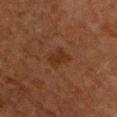This lesion was catalogued during total-body skin photography and was not selected for biopsy.
An algorithmic analysis of the crop reported an area of roughly 5 mm² and two-axis asymmetry of about 0.2. The analysis additionally found a lesion color around L≈23 a*≈17 b*≈25 in CIELAB and a normalized border contrast of about 6.5. It also reported a border-irregularity rating of about 2.5/10, a color-variation rating of about 1.5/10, and radial color variation of about 0.5.
The tile uses cross-polarized illumination.
The recorded lesion diameter is about 3 mm.
From the chest.
A male patient aged around 60.
A roughly 15 mm field-of-view crop from a total-body skin photograph.